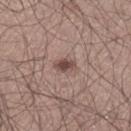{
  "biopsy_status": "not biopsied; imaged during a skin examination",
  "automated_metrics": {
    "area_mm2_approx": 3.5,
    "eccentricity": 0.8,
    "shape_asymmetry": 0.2,
    "vs_skin_darker_L": 12.0,
    "lesion_detection_confidence_0_100": 100
  },
  "site": "left thigh",
  "lesion_size": {
    "long_diameter_mm_approx": 2.5
  },
  "lighting": "white-light",
  "patient": {
    "sex": "male",
    "age_approx": 65
  },
  "image": {
    "source": "total-body photography crop",
    "field_of_view_mm": 15
  }
}